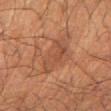| feature | finding |
|---|---|
| automated metrics | an average lesion color of about L≈37 a*≈19 b*≈26 (CIELAB), a lesion–skin lightness drop of about 6, and a normalized lesion–skin contrast near 5.5; radial color variation of about 0.5 |
| body site | the arm |
| patient | male, approximately 60 years of age |
| image source | ~15 mm crop, total-body skin-cancer survey |